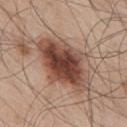Clinical impression:
Imaged during a routine full-body skin examination; the lesion was not biopsied and no histopathology is available.
Acquisition and patient details:
This image is a 15 mm lesion crop taken from a total-body photograph. A male subject about 55 years old. The recorded lesion diameter is about 8.5 mm. From the upper back. The total-body-photography lesion software estimated an average lesion color of about L≈45 a*≈21 b*≈26 (CIELAB) and roughly 17 lightness units darker than nearby skin. The tile uses white-light illumination.